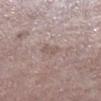Q: Is there a histopathology result?
A: imaged on a skin check; not biopsied
Q: Patient demographics?
A: male, in their mid-70s
Q: What is the imaging modality?
A: ~15 mm crop, total-body skin-cancer survey
Q: What lighting was used for the tile?
A: white-light
Q: How large is the lesion?
A: about 2.5 mm
Q: What did automated image analysis measure?
A: a mean CIELAB color near L≈56 a*≈15 b*≈20 and a normalized border contrast of about 4.5; a border-irregularity rating of about 2.5/10, a within-lesion color-variation index near 1.5/10, and radial color variation of about 0.5
Q: What is the anatomic site?
A: the leg